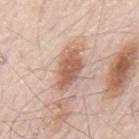The lesion was photographed on a routine skin check and not biopsied; there is no pathology result.
A male subject, about 80 years old.
A 15 mm close-up extracted from a 3D total-body photography capture.
Captured under white-light illumination.
From the mid back.
The recorded lesion diameter is about 5 mm.